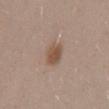notes = imaged on a skin check; not biopsied
anatomic site = the mid back
image = ~15 mm crop, total-body skin-cancer survey
size = ≈3 mm
patient = male, aged 28 to 32